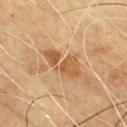{
  "biopsy_status": "not biopsied; imaged during a skin examination",
  "image": {
    "source": "total-body photography crop",
    "field_of_view_mm": 15
  },
  "patient": {
    "sex": "male",
    "age_approx": 65
  },
  "lighting": "cross-polarized",
  "lesion_size": {
    "long_diameter_mm_approx": 5.5
  },
  "site": "chest"
}